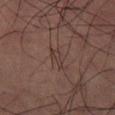Clinical summary:
The patient is a male aged around 60. Cropped from a whole-body photographic skin survey; the tile spans about 15 mm. The lesion's longest dimension is about 2.5 mm.